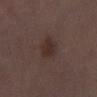- biopsy status · catalogued during a skin exam; not biopsied
- tile lighting · white-light illumination
- image source · ~15 mm crop, total-body skin-cancer survey
- subject · male, aged 68 to 72
- anatomic site · the left lower leg
- automated lesion analysis · a border-irregularity index near 1.5/10, internal color variation of about 2 on a 0–10 scale, and peripheral color asymmetry of about 0.5; a detector confidence of about 100 out of 100 that the crop contains a lesion